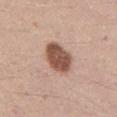Imaged during a routine full-body skin examination; the lesion was not biopsied and no histopathology is available. A male subject, aged 33–37. Approximately 4.5 mm at its widest. A close-up tile cropped from a whole-body skin photograph, about 15 mm across. The lesion is located on the mid back. Imaged with white-light lighting.